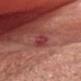Impression:
The lesion was photographed on a routine skin check and not biopsied; there is no pathology result.